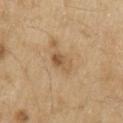Findings:
– notes: total-body-photography surveillance lesion; no biopsy
– tile lighting: white-light
– acquisition: ~15 mm tile from a whole-body skin photo
– subject: male, approximately 70 years of age
– site: the arm